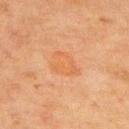<tbp_lesion>
<biopsy_status>not biopsied; imaged during a skin examination</biopsy_status>
<automated_metrics>
  <nevus_likeness_0_100>5</nevus_likeness_0_100>
  <lesion_detection_confidence_0_100>100</lesion_detection_confidence_0_100>
</automated_metrics>
<patient>
  <sex>male</sex>
  <age_approx>70</age_approx>
</patient>
<lesion_size>
  <long_diameter_mm_approx>4.0</long_diameter_mm_approx>
</lesion_size>
<site>upper back</site>
<image>
  <source>total-body photography crop</source>
  <field_of_view_mm>15</field_of_view_mm>
</image>
<lighting>cross-polarized</lighting>
</tbp_lesion>